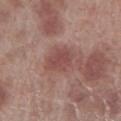Case summary:
* anatomic site: the right lower leg
* diameter: ~3.5 mm (longest diameter)
* acquisition: ~15 mm tile from a whole-body skin photo
* patient: male, aged approximately 70
* illumination: white-light illumination